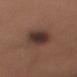Assessment: The lesion was photographed on a routine skin check and not biopsied; there is no pathology result. Clinical summary: Longest diameter approximately 4 mm. On the left upper arm. A close-up tile cropped from a whole-body skin photograph, about 15 mm across. The patient is a male aged around 60. The tile uses white-light illumination.